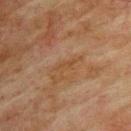follow-up: catalogued during a skin exam; not biopsied | body site: the upper back | lighting: cross-polarized | image: total-body-photography crop, ~15 mm field of view | patient: male, aged around 75 | image-analysis metrics: a footprint of about 2.5 mm², an outline eccentricity of about 0.9 (0 = round, 1 = elongated), and two-axis asymmetry of about 0.4; an average lesion color of about L≈39 a*≈17 b*≈30 (CIELAB) and roughly 4 lightness units darker than nearby skin; an automated nevus-likeness rating near 0 out of 100 | lesion diameter: ≈2.5 mm.